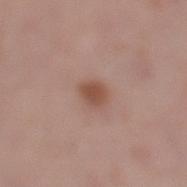site=the left lower leg
patient=female, aged 48–52
image-analysis metrics=an area of roughly 4.5 mm²; a lesion color around L≈50 a*≈21 b*≈27 in CIELAB, a lesion–skin lightness drop of about 10, and a normalized lesion–skin contrast near 8; internal color variation of about 1.5 on a 0–10 scale and radial color variation of about 0.5; a nevus-likeness score of about 90/100 and a lesion-detection confidence of about 100/100
tile lighting=white-light illumination
diameter=~2.5 mm (longest diameter)
imaging modality=total-body-photography crop, ~15 mm field of view